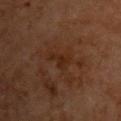Captured during whole-body skin photography for melanoma surveillance; the lesion was not biopsied. Captured under cross-polarized illumination. Automated image analysis of the tile measured border irregularity of about 6 on a 0–10 scale, a color-variation rating of about 1/10, and peripheral color asymmetry of about 0.5. From the chest. A male patient, aged approximately 60. A lesion tile, about 15 mm wide, cut from a 3D total-body photograph.